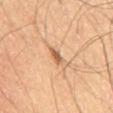Assessment:
No biopsy was performed on this lesion — it was imaged during a full skin examination and was not determined to be concerning.
Acquisition and patient details:
From the abdomen. Imaged with cross-polarized lighting. This image is a 15 mm lesion crop taken from a total-body photograph. A male subject, roughly 55 years of age.